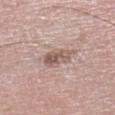Assessment:
Part of a total-body skin-imaging series; this lesion was reviewed on a skin check and was not flagged for biopsy.
Image and clinical context:
A region of skin cropped from a whole-body photographic capture, roughly 15 mm wide. The recorded lesion diameter is about 4 mm. The lesion is located on the left lower leg. Automated image analysis of the tile measured a lesion area of about 7.5 mm², an eccentricity of roughly 0.75, and a symmetry-axis asymmetry near 0.2. The analysis additionally found about 11 CIELAB-L* units darker than the surrounding skin and a normalized lesion–skin contrast near 7. And it measured a nevus-likeness score of about 40/100 and lesion-presence confidence of about 100/100. A male subject, about 75 years old. This is a white-light tile.